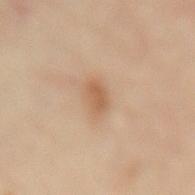The lesion was photographed on a routine skin check and not biopsied; there is no pathology result. A female patient, roughly 60 years of age. This image is a 15 mm lesion crop taken from a total-body photograph. This is a cross-polarized tile. The lesion-visualizer software estimated a shape-asymmetry score of about 0.2 (0 = symmetric). And it measured border irregularity of about 2 on a 0–10 scale, a color-variation rating of about 1/10, and a peripheral color-asymmetry measure near 0.5. Longest diameter approximately 3 mm. Located on the lower back.